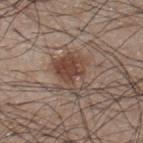The lesion was photographed on a routine skin check and not biopsied; there is no pathology result. Located on the back. About 5 mm across. A 15 mm crop from a total-body photograph taken for skin-cancer surveillance. A male patient, aged 43–47. Automated image analysis of the tile measured an eccentricity of roughly 0.65 and a symmetry-axis asymmetry near 0.4. It also reported roughly 10 lightness units darker than nearby skin. The software also gave border irregularity of about 4.5 on a 0–10 scale, a within-lesion color-variation index near 6.5/10, and peripheral color asymmetry of about 2.5.